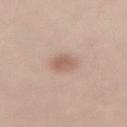Imaged during a routine full-body skin examination; the lesion was not biopsied and no histopathology is available.
On the lower back.
A 15 mm close-up extracted from a 3D total-body photography capture.
The tile uses white-light illumination.
A female subject, roughly 40 years of age.